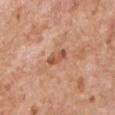Q: Was a biopsy performed?
A: catalogued during a skin exam; not biopsied
Q: Lesion location?
A: the chest
Q: Patient demographics?
A: male, approximately 60 years of age
Q: What is the imaging modality?
A: ~15 mm tile from a whole-body skin photo
Q: How large is the lesion?
A: ≈3 mm
Q: What did automated image analysis measure?
A: border irregularity of about 3.5 on a 0–10 scale, a color-variation rating of about 0/10, and radial color variation of about 0; a classifier nevus-likeness of about 0/100 and a lesion-detection confidence of about 100/100
Q: How was the tile lit?
A: white-light illumination